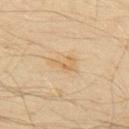Q: Was this lesion biopsied?
A: no biopsy performed (imaged during a skin exam)
Q: What is the lesion's diameter?
A: about 3.5 mm
Q: Lesion location?
A: the upper back
Q: Automated lesion metrics?
A: a lesion–skin lightness drop of about 6 and a normalized lesion–skin contrast near 5.5; internal color variation of about 3.5 on a 0–10 scale and peripheral color asymmetry of about 1.5; a classifier nevus-likeness of about 0/100 and a lesion-detection confidence of about 100/100
Q: Patient demographics?
A: male, in their mid-30s
Q: How was this image acquired?
A: ~15 mm tile from a whole-body skin photo
Q: Illumination type?
A: cross-polarized illumination